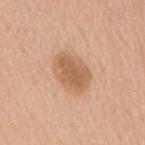Assessment: The lesion was photographed on a routine skin check and not biopsied; there is no pathology result. Clinical summary: Imaged with white-light lighting. On the back. A lesion tile, about 15 mm wide, cut from a 3D total-body photograph. The subject is a female aged approximately 60. Approximately 5 mm at its widest.